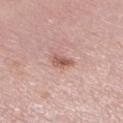Q: Was this lesion biopsied?
A: catalogued during a skin exam; not biopsied
Q: Automated lesion metrics?
A: an average lesion color of about L≈57 a*≈23 b*≈26 (CIELAB) and a lesion-to-skin contrast of about 7.5 (normalized; higher = more distinct); a border-irregularity index near 3.5/10 and peripheral color asymmetry of about 1; an automated nevus-likeness rating near 45 out of 100
Q: What are the patient's age and sex?
A: female, in their 40s
Q: Lesion location?
A: the right lower leg
Q: What is the lesion's diameter?
A: about 3 mm
Q: What kind of image is this?
A: ~15 mm crop, total-body skin-cancer survey